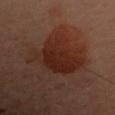biopsy status = catalogued during a skin exam; not biopsied
subject = female, approximately 60 years of age
acquisition = 15 mm crop, total-body photography
body site = the back